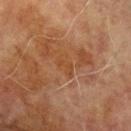Image and clinical context:
This is a cross-polarized tile. A close-up tile cropped from a whole-body skin photograph, about 15 mm across. A male patient aged 68 to 72. Automated tile analysis of the lesion measured an area of roughly 3.5 mm², an outline eccentricity of about 0.8 (0 = round, 1 = elongated), and a shape-asymmetry score of about 0.4 (0 = symmetric). The analysis additionally found a border-irregularity rating of about 4/10, a within-lesion color-variation index near 0.5/10, and a peripheral color-asymmetry measure near 0. From the left upper arm. The recorded lesion diameter is about 2.5 mm.